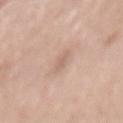  biopsy_status: not biopsied; imaged during a skin examination
  site: mid back
  image:
    source: total-body photography crop
    field_of_view_mm: 15
  lighting: white-light
  lesion_size:
    long_diameter_mm_approx: 3.0
  patient:
    sex: female
    age_approx: 40
  automated_metrics:
    color_variation_0_10: 0.5
    peripheral_color_asymmetry: 0.0
    nevus_likeness_0_100: 0
    lesion_detection_confidence_0_100: 100